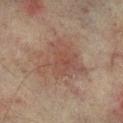| field | value |
|---|---|
| notes | no biopsy performed (imaged during a skin exam) |
| site | the right lower leg |
| imaging modality | ~15 mm tile from a whole-body skin photo |
| diameter | ~6 mm (longest diameter) |
| subject | male, aged approximately 75 |
| TBP lesion metrics | a border-irregularity rating of about 5.5/10, a color-variation rating of about 3/10, and radial color variation of about 1 |